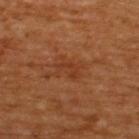Clinical impression:
The lesion was photographed on a routine skin check and not biopsied; there is no pathology result.
Image and clinical context:
A lesion tile, about 15 mm wide, cut from a 3D total-body photograph. Captured under cross-polarized illumination. Approximately 3 mm at its widest. The subject is a male aged approximately 65. From the upper back.